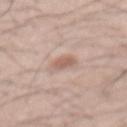– biopsy status: catalogued during a skin exam; not biopsied
– patient: male, roughly 55 years of age
– location: the mid back
– image-analysis metrics: a shape-asymmetry score of about 0.2 (0 = symmetric); border irregularity of about 2 on a 0–10 scale, internal color variation of about 1.5 on a 0–10 scale, and radial color variation of about 0.5; an automated nevus-likeness rating near 65 out of 100 and a detector confidence of about 100 out of 100 that the crop contains a lesion
– imaging modality: 15 mm crop, total-body photography
– lesion size: ~2.5 mm (longest diameter)